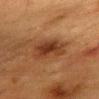The lesion was tiled from a total-body skin photograph and was not biopsied. This is a cross-polarized tile. A region of skin cropped from a whole-body photographic capture, roughly 15 mm wide. The patient is a female roughly 55 years of age. Automated image analysis of the tile measured a mean CIELAB color near L≈31 a*≈20 b*≈29, about 9 CIELAB-L* units darker than the surrounding skin, and a normalized lesion–skin contrast near 8.5. The lesion is on the chest.